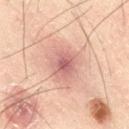Clinical impression: Captured during whole-body skin photography for melanoma surveillance; the lesion was not biopsied. Context: This is a cross-polarized tile. A male patient, about 50 years old. The lesion is on the right thigh. A region of skin cropped from a whole-body photographic capture, roughly 15 mm wide. Automated image analysis of the tile measured a footprint of about 18 mm² and an outline eccentricity of about 0.7 (0 = round, 1 = elongated). It also reported roughly 9 lightness units darker than nearby skin. The analysis additionally found a border-irregularity rating of about 3/10 and internal color variation of about 5 on a 0–10 scale. The analysis additionally found lesion-presence confidence of about 100/100. Approximately 5.5 mm at its widest.